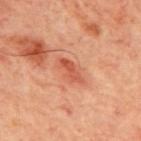{
  "biopsy_status": "not biopsied; imaged during a skin examination"
}